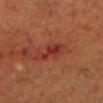Q: Was this lesion biopsied?
A: total-body-photography surveillance lesion; no biopsy
Q: Patient demographics?
A: male, aged 63 to 67
Q: How was the tile lit?
A: cross-polarized
Q: What is the lesion's diameter?
A: ~4.5 mm (longest diameter)
Q: Where on the body is the lesion?
A: the head or neck
Q: What kind of image is this?
A: ~15 mm tile from a whole-body skin photo
Q: What did automated image analysis measure?
A: a lesion color around L≈35 a*≈32 b*≈28 in CIELAB, about 8 CIELAB-L* units darker than the surrounding skin, and a normalized border contrast of about 7.5; border irregularity of about 5 on a 0–10 scale; a nevus-likeness score of about 0/100 and lesion-presence confidence of about 95/100